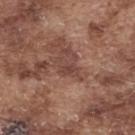The lesion was photographed on a routine skin check and not biopsied; there is no pathology result. The lesion is on the upper back. A 15 mm close-up tile from a total-body photography series done for melanoma screening. The lesion's longest dimension is about 3 mm. The tile uses white-light illumination. A male patient aged 73–77.